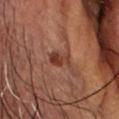Impression:
Part of a total-body skin-imaging series; this lesion was reviewed on a skin check and was not flagged for biopsy.
Clinical summary:
About 2.5 mm across. This image is a 15 mm lesion crop taken from a total-body photograph. The patient is a male aged 68 to 72. Captured under cross-polarized illumination. An algorithmic analysis of the crop reported a mean CIELAB color near L≈37 a*≈24 b*≈28, roughly 10 lightness units darker than nearby skin, and a normalized lesion–skin contrast near 8.5. Located on the head or neck.